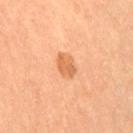{"biopsy_status": "not biopsied; imaged during a skin examination", "image": {"source": "total-body photography crop", "field_of_view_mm": 15}, "lesion_size": {"long_diameter_mm_approx": 3.5}, "lighting": "cross-polarized", "patient": {"sex": "female", "age_approx": 65}, "site": "left leg"}